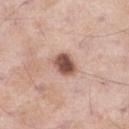Imaged during a routine full-body skin examination; the lesion was not biopsied and no histopathology is available.
Located on the left thigh.
A male patient in their mid-50s.
Cropped from a whole-body photographic skin survey; the tile spans about 15 mm.
Automated image analysis of the tile measured border irregularity of about 1.5 on a 0–10 scale, internal color variation of about 5 on a 0–10 scale, and peripheral color asymmetry of about 1.5.